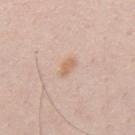biopsy status: total-body-photography surveillance lesion; no biopsy | patient: male, about 35 years old | image source: ~15 mm crop, total-body skin-cancer survey | location: the mid back | tile lighting: white-light.